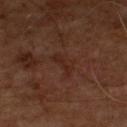Assessment: The lesion was tiled from a total-body skin photograph and was not biopsied. Acquisition and patient details: The subject is a male in their mid- to late 50s. Automated tile analysis of the lesion measured a footprint of about 5.5 mm², an outline eccentricity of about 0.8 (0 = round, 1 = elongated), and a symmetry-axis asymmetry near 0.4. The software also gave a border-irregularity rating of about 4.5/10 and a within-lesion color-variation index near 2.5/10. Approximately 3.5 mm at its widest. A 15 mm close-up extracted from a 3D total-body photography capture. On the chest.